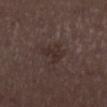The lesion was tiled from a total-body skin photograph and was not biopsied.
The lesion-visualizer software estimated an outline eccentricity of about 0.6 (0 = round, 1 = elongated) and a symmetry-axis asymmetry near 0.5. The software also gave about 5 CIELAB-L* units darker than the surrounding skin and a lesion-to-skin contrast of about 6 (normalized; higher = more distinct). The software also gave a nevus-likeness score of about 0/100.
This is a white-light tile.
The lesion is located on the left lower leg.
A roughly 15 mm field-of-view crop from a total-body skin photograph.
Approximately 2.5 mm at its widest.
A female patient, aged 48 to 52.